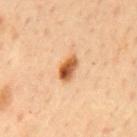Impression: The lesion was photographed on a routine skin check and not biopsied; there is no pathology result. Clinical summary: A male subject, aged 53–57. Approximately 3.5 mm at its widest. This is a cross-polarized tile. Automated image analysis of the tile measured an automated nevus-likeness rating near 100 out of 100 and a detector confidence of about 100 out of 100 that the crop contains a lesion. The lesion is located on the mid back. Cropped from a whole-body photographic skin survey; the tile spans about 15 mm.